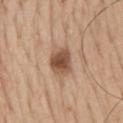Automated image analysis of the tile measured an area of roughly 7.5 mm² and a symmetry-axis asymmetry near 0.2. And it measured an average lesion color of about L≈51 a*≈20 b*≈31 (CIELAB) and a normalized lesion–skin contrast near 9.5. The analysis additionally found border irregularity of about 1.5 on a 0–10 scale, a within-lesion color-variation index near 4/10, and radial color variation of about 1. And it measured a classifier nevus-likeness of about 90/100. A male patient, approximately 65 years of age. A close-up tile cropped from a whole-body skin photograph, about 15 mm across. About 3.5 mm across. From the mid back.